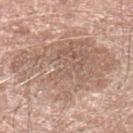Impression: Imaged during a routine full-body skin examination; the lesion was not biopsied and no histopathology is available. Clinical summary: This is a white-light tile. The lesion is located on the left lower leg. A region of skin cropped from a whole-body photographic capture, roughly 15 mm wide. The patient is a male aged around 80.